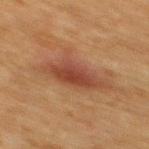Assessment: Imaged during a routine full-body skin examination; the lesion was not biopsied and no histopathology is available. Acquisition and patient details: The total-body-photography lesion software estimated a lesion color around L≈36 a*≈21 b*≈27 in CIELAB and a normalized border contrast of about 7.5. It also reported an automated nevus-likeness rating near 70 out of 100 and a detector confidence of about 100 out of 100 that the crop contains a lesion. The lesion is on the back. A male subject, aged 48–52. Cropped from a total-body skin-imaging series; the visible field is about 15 mm. Imaged with cross-polarized lighting. Approximately 6 mm at its widest.